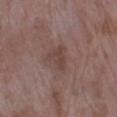| feature | finding |
|---|---|
| biopsy status | catalogued during a skin exam; not biopsied |
| imaging modality | total-body-photography crop, ~15 mm field of view |
| body site | the left lower leg |
| diameter | about 3 mm |
| subject | female, approximately 70 years of age |
| TBP lesion metrics | an area of roughly 5 mm² and an outline eccentricity of about 0.65 (0 = round, 1 = elongated); an average lesion color of about L≈42 a*≈18 b*≈21 (CIELAB), about 7 CIELAB-L* units darker than the surrounding skin, and a normalized border contrast of about 6 |